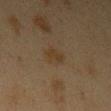Q: Was this lesion biopsied?
A: catalogued during a skin exam; not biopsied
Q: Lesion location?
A: the arm
Q: Patient demographics?
A: female, aged 38 to 42
Q: What is the imaging modality?
A: 15 mm crop, total-body photography